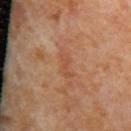No biopsy was performed on this lesion — it was imaged during a full skin examination and was not determined to be concerning.
A lesion tile, about 15 mm wide, cut from a 3D total-body photograph.
From the mid back.
The patient is a female roughly 55 years of age.
Automated image analysis of the tile measured a nevus-likeness score of about 0/100 and lesion-presence confidence of about 95/100.
Longest diameter approximately 3 mm.